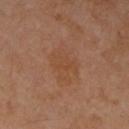Findings:
– biopsy status — total-body-photography surveillance lesion; no biopsy
– size — about 5 mm
– acquisition — total-body-photography crop, ~15 mm field of view
– anatomic site — the right upper arm
– subject — female, about 55 years old
– automated lesion analysis — a lesion area of about 13 mm², an eccentricity of roughly 0.7, and a symmetry-axis asymmetry near 0.2; about 4 CIELAB-L* units darker than the surrounding skin and a normalized border contrast of about 4.5; border irregularity of about 2.5 on a 0–10 scale and a peripheral color-asymmetry measure near 1.5; a classifier nevus-likeness of about 0/100 and lesion-presence confidence of about 100/100